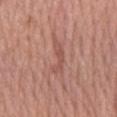Assessment:
Part of a total-body skin-imaging series; this lesion was reviewed on a skin check and was not flagged for biopsy.
Context:
A 15 mm crop from a total-body photograph taken for skin-cancer surveillance. A female patient aged around 60. This is a white-light tile. An algorithmic analysis of the crop reported an eccentricity of roughly 0.95 and two-axis asymmetry of about 0.4. The software also gave a mean CIELAB color near L≈53 a*≈23 b*≈26, a lesion–skin lightness drop of about 7, and a lesion-to-skin contrast of about 5 (normalized; higher = more distinct). It also reported a nevus-likeness score of about 0/100 and lesion-presence confidence of about 60/100. Located on the left forearm.